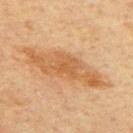biopsy status = catalogued during a skin exam; not biopsied
imaging modality = 15 mm crop, total-body photography
site = the upper back
patient = male, aged approximately 65